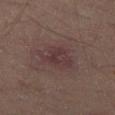Q: Was this lesion biopsied?
A: no biopsy performed (imaged during a skin exam)
Q: Where on the body is the lesion?
A: the left thigh
Q: What is the imaging modality?
A: ~15 mm crop, total-body skin-cancer survey
Q: How was the tile lit?
A: cross-polarized illumination
Q: What did automated image analysis measure?
A: an area of roughly 10 mm², an outline eccentricity of about 0.7 (0 = round, 1 = elongated), and two-axis asymmetry of about 0.35; a mean CIELAB color near L≈30 a*≈15 b*≈14, about 5 CIELAB-L* units darker than the surrounding skin, and a normalized lesion–skin contrast near 6; a border-irregularity index near 4.5/10, a color-variation rating of about 3/10, and peripheral color asymmetry of about 1
Q: How large is the lesion?
A: ≈5 mm
Q: Patient demographics?
A: male, aged 48 to 52